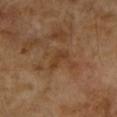subject=female, roughly 70 years of age | site=the left forearm | lesion size=≈3 mm | automated metrics=border irregularity of about 4.5 on a 0–10 scale and radial color variation of about 0; a classifier nevus-likeness of about 0/100 and lesion-presence confidence of about 100/100 | tile lighting=cross-polarized | image=~15 mm crop, total-body skin-cancer survey.